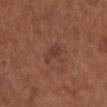Assessment: Part of a total-body skin-imaging series; this lesion was reviewed on a skin check and was not flagged for biopsy. Image and clinical context: The recorded lesion diameter is about 3 mm. A female subject roughly 55 years of age. Cropped from a whole-body photographic skin survey; the tile spans about 15 mm. On the right lower leg.